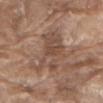Notes:
– workup: catalogued during a skin exam; not biopsied
– acquisition: ~15 mm tile from a whole-body skin photo
– lesion size: about 7 mm
– subject: male, aged approximately 80
– body site: the back